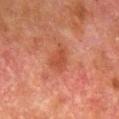Assessment:
No biopsy was performed on this lesion — it was imaged during a full skin examination and was not determined to be concerning.
Clinical summary:
This is a cross-polarized tile. From the leg. A 15 mm close-up extracted from a 3D total-body photography capture. The patient is a male aged approximately 80.